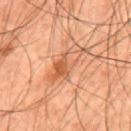This lesion was catalogued during total-body skin photography and was not selected for biopsy.
This is a cross-polarized tile.
A close-up tile cropped from a whole-body skin photograph, about 15 mm across.
On the mid back.
A male subject, aged 43–47.
Longest diameter approximately 5.5 mm.